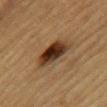<tbp_lesion>
  <biopsy_status>not biopsied; imaged during a skin examination</biopsy_status>
  <image>
    <source>total-body photography crop</source>
    <field_of_view_mm>15</field_of_view_mm>
  </image>
  <patient>
    <sex>male</sex>
    <age_approx>85+</age_approx>
  </patient>
  <site>left upper arm</site>
  <lighting>cross-polarized</lighting>
  <lesion_size>
    <long_diameter_mm_approx>4.0</long_diameter_mm_approx>
  </lesion_size>
</tbp_lesion>